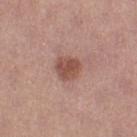patient:
  sex: female
  age_approx: 35
automated_metrics:
  eccentricity: 0.35
  nevus_likeness_0_100: 70
image:
  source: total-body photography crop
  field_of_view_mm: 15
site: left thigh
lighting: white-light
lesion_size:
  long_diameter_mm_approx: 3.0